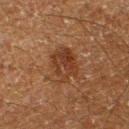Captured during whole-body skin photography for melanoma surveillance; the lesion was not biopsied. The lesion is located on the left lower leg. The subject is a male aged around 60. This image is a 15 mm lesion crop taken from a total-body photograph.